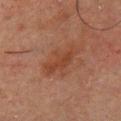* workup: imaged on a skin check; not biopsied
* location: the chest
* illumination: cross-polarized
* TBP lesion metrics: a lesion area of about 9.5 mm² and two-axis asymmetry of about 0.25; a lesion color around L≈33 a*≈19 b*≈26 in CIELAB, roughly 6 lightness units darker than nearby skin, and a lesion-to-skin contrast of about 6.5 (normalized; higher = more distinct); border irregularity of about 4.5 on a 0–10 scale, a within-lesion color-variation index near 1.5/10, and radial color variation of about 0.5
* imaging modality: ~15 mm tile from a whole-body skin photo
* subject: male, approximately 50 years of age